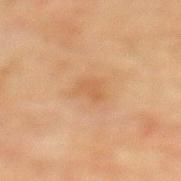{
  "biopsy_status": "not biopsied; imaged during a skin examination",
  "patient": {
    "sex": "female",
    "age_approx": 80
  },
  "site": "mid back",
  "image": {
    "source": "total-body photography crop",
    "field_of_view_mm": 15
  }
}